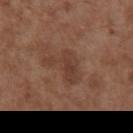Q: Was this lesion biopsied?
A: catalogued during a skin exam; not biopsied
Q: Lesion size?
A: ≈4.5 mm
Q: What is the imaging modality?
A: ~15 mm crop, total-body skin-cancer survey
Q: What lighting was used for the tile?
A: white-light
Q: What are the patient's age and sex?
A: male, aged 73–77
Q: Where on the body is the lesion?
A: the chest